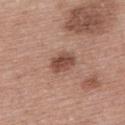* workup — catalogued during a skin exam; not biopsied
* subject — female, aged around 50
* acquisition — ~15 mm tile from a whole-body skin photo
* body site — the upper back